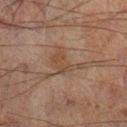workup: imaged on a skin check; not biopsied | patient: male, approximately 60 years of age | TBP lesion metrics: a nevus-likeness score of about 0/100 | lesion diameter: ~3.5 mm (longest diameter) | location: the left leg | acquisition: 15 mm crop, total-body photography | illumination: cross-polarized.